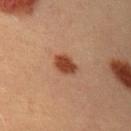Clinical impression: This lesion was catalogued during total-body skin photography and was not selected for biopsy. Acquisition and patient details: Measured at roughly 3 mm in maximum diameter. This is a cross-polarized tile. The patient is a male about 40 years old. Located on the right upper arm. A 15 mm crop from a total-body photograph taken for skin-cancer surveillance.